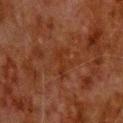| field | value |
|---|---|
| notes | imaged on a skin check; not biopsied |
| patient | male, in their 80s |
| image | ~15 mm crop, total-body skin-cancer survey |
| location | the back |